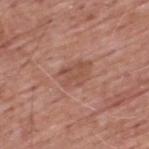Clinical impression: No biopsy was performed on this lesion — it was imaged during a full skin examination and was not determined to be concerning. Acquisition and patient details: The tile uses white-light illumination. Approximately 4.5 mm at its widest. The total-body-photography lesion software estimated a lesion area of about 9 mm², an outline eccentricity of about 0.7 (0 = round, 1 = elongated), and two-axis asymmetry of about 0.2. The software also gave a border-irregularity rating of about 2/10, a within-lesion color-variation index near 3/10, and radial color variation of about 1. Located on the back. A male patient in their 60s. A lesion tile, about 15 mm wide, cut from a 3D total-body photograph.